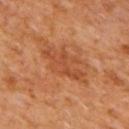follow-up=catalogued during a skin exam; not biopsied | location=the mid back | image source=~15 mm tile from a whole-body skin photo | patient=male, aged 63 to 67.